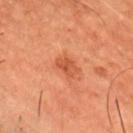Findings:
* biopsy status: total-body-photography surveillance lesion; no biopsy
* patient: male, about 50 years old
* acquisition: total-body-photography crop, ~15 mm field of view
* body site: the chest
* lighting: cross-polarized illumination
* diameter: about 2.5 mm